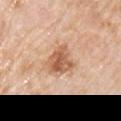biopsy_status: not biopsied; imaged during a skin examination
patient:
  sex: male
  age_approx: 75
lesion_size:
  long_diameter_mm_approx: 4.0
image:
  source: total-body photography crop
  field_of_view_mm: 15
lighting: white-light
site: right upper arm